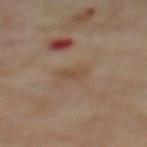The lesion was photographed on a routine skin check and not biopsied; there is no pathology result.
A female subject aged 58 to 62.
About 2.5 mm across.
The lesion is located on the back.
Captured under cross-polarized illumination.
A roughly 15 mm field-of-view crop from a total-body skin photograph.